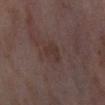<case>
  <biopsy_status>not biopsied; imaged during a skin examination</biopsy_status>
  <lighting>cross-polarized</lighting>
  <image>
    <source>total-body photography crop</source>
    <field_of_view_mm>15</field_of_view_mm>
  </image>
  <automated_metrics>
    <cielab_L>33</cielab_L>
    <cielab_a>16</cielab_a>
    <cielab_b>19</cielab_b>
    <vs_skin_contrast_norm>5.5</vs_skin_contrast_norm>
    <border_irregularity_0_10>4.5</border_irregularity_0_10>
    <peripheral_color_asymmetry>0.5</peripheral_color_asymmetry>
    <lesion_detection_confidence_0_100>100</lesion_detection_confidence_0_100>
  </automated_metrics>
  <patient>
    <sex>female</sex>
    <age_approx>55</age_approx>
  </patient>
  <site>right thigh</site>
</case>